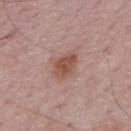workup — imaged on a skin check; not biopsied
body site — the mid back
diameter — ~3.5 mm (longest diameter)
TBP lesion metrics — a shape eccentricity near 0.75 and a symmetry-axis asymmetry near 0.2; a mean CIELAB color near L≈51 a*≈22 b*≈26 and a lesion–skin lightness drop of about 10; a border-irregularity index near 2/10, a within-lesion color-variation index near 3.5/10, and a peripheral color-asymmetry measure near 1; a classifier nevus-likeness of about 75/100 and a lesion-detection confidence of about 100/100
tile lighting — white-light illumination
patient — male, in their 50s
acquisition — total-body-photography crop, ~15 mm field of view